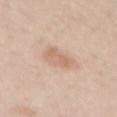workup = imaged on a skin check; not biopsied | lesion size = ≈4.5 mm | imaging modality = ~15 mm crop, total-body skin-cancer survey | subject = female, aged 43 to 47 | anatomic site = the mid back | lighting = white-light illumination.